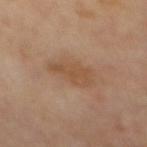Notes:
– workup · imaged on a skin check; not biopsied
– imaging modality · ~15 mm tile from a whole-body skin photo
– location · the mid back